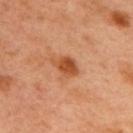{
  "biopsy_status": "not biopsied; imaged during a skin examination",
  "patient": {
    "sex": "male",
    "age_approx": 50
  },
  "site": "upper back",
  "lighting": "cross-polarized",
  "automated_metrics": {
    "eccentricity": 0.7,
    "shape_asymmetry": 0.2,
    "nevus_likeness_0_100": 75
  },
  "lesion_size": {
    "long_diameter_mm_approx": 3.0
  },
  "image": {
    "source": "total-body photography crop",
    "field_of_view_mm": 15
  }
}